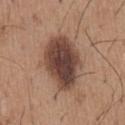illumination: white-light; subject: male, in their mid- to late 60s; image source: ~15 mm crop, total-body skin-cancer survey; size: ≈6.5 mm; site: the chest.